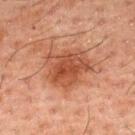Part of a total-body skin-imaging series; this lesion was reviewed on a skin check and was not flagged for biopsy. The lesion is on the upper back. The recorded lesion diameter is about 5.5 mm. Automated tile analysis of the lesion measured a lesion area of about 16 mm² and an eccentricity of roughly 0.65. The analysis additionally found roughly 10 lightness units darker than nearby skin. Imaged with cross-polarized lighting. The subject is a male aged around 50. A close-up tile cropped from a whole-body skin photograph, about 15 mm across.